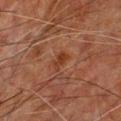Notes:
– workup · total-body-photography surveillance lesion; no biopsy
– tile lighting · cross-polarized
– image source · 15 mm crop, total-body photography
– size · ~2.5 mm (longest diameter)
– body site · the chest
– subject · male, about 65 years old